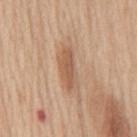Part of a total-body skin-imaging series; this lesion was reviewed on a skin check and was not flagged for biopsy. The lesion is located on the mid back. A 15 mm close-up tile from a total-body photography series done for melanoma screening. A male patient approximately 60 years of age. Captured under white-light illumination.